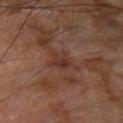Case summary:
• workup — no biopsy performed (imaged during a skin exam)
• site — the arm
• diameter — ~2.5 mm (longest diameter)
• patient — male, about 65 years old
• imaging modality — ~15 mm crop, total-body skin-cancer survey
• lighting — cross-polarized